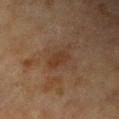biopsy status = catalogued during a skin exam; not biopsied | body site = the left forearm | size = ~2.5 mm (longest diameter) | subject = female, aged approximately 80 | illumination = cross-polarized | image source = 15 mm crop, total-body photography.